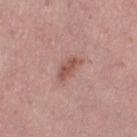Impression: No biopsy was performed on this lesion — it was imaged during a full skin examination and was not determined to be concerning. Acquisition and patient details: The lesion is on the right thigh. Approximately 3.5 mm at its widest. A female subject aged around 40. A 15 mm close-up tile from a total-body photography series done for melanoma screening.